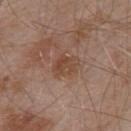Q: Was a biopsy performed?
A: total-body-photography surveillance lesion; no biopsy
Q: Patient demographics?
A: male, aged around 55
Q: What did automated image analysis measure?
A: a lesion–skin lightness drop of about 7 and a normalized lesion–skin contrast near 6.5; a border-irregularity rating of about 2.5/10, internal color variation of about 3.5 on a 0–10 scale, and radial color variation of about 1; a classifier nevus-likeness of about 0/100 and lesion-presence confidence of about 100/100
Q: What is the anatomic site?
A: the upper back
Q: Illumination type?
A: white-light illumination
Q: What is the imaging modality?
A: 15 mm crop, total-body photography
Q: Lesion size?
A: about 3 mm